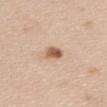Q: Was this lesion biopsied?
A: catalogued during a skin exam; not biopsied
Q: What is the lesion's diameter?
A: ~3 mm (longest diameter)
Q: How was this image acquired?
A: ~15 mm crop, total-body skin-cancer survey
Q: Who is the patient?
A: female, approximately 50 years of age
Q: Automated lesion metrics?
A: a footprint of about 4 mm² and a shape eccentricity near 0.8; a border-irregularity rating of about 2.5/10, internal color variation of about 4.5 on a 0–10 scale, and peripheral color asymmetry of about 1.5; a nevus-likeness score of about 95/100 and a lesion-detection confidence of about 100/100
Q: Where on the body is the lesion?
A: the upper back
Q: Illumination type?
A: white-light illumination